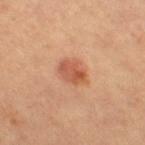Clinical impression:
The lesion was tiled from a total-body skin photograph and was not biopsied.
Context:
A female patient roughly 60 years of age. Automated tile analysis of the lesion measured an average lesion color of about L≈58 a*≈28 b*≈36 (CIELAB), a lesion–skin lightness drop of about 11, and a normalized lesion–skin contrast near 7. The software also gave a detector confidence of about 100 out of 100 that the crop contains a lesion. From the right thigh. Cropped from a whole-body photographic skin survey; the tile spans about 15 mm. Measured at roughly 3.5 mm in maximum diameter. Imaged with cross-polarized lighting.